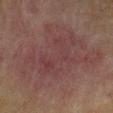Clinical impression:
This lesion was catalogued during total-body skin photography and was not selected for biopsy.
Acquisition and patient details:
The tile uses cross-polarized illumination. On the right upper arm. A roughly 15 mm field-of-view crop from a total-body skin photograph. The lesion's longest dimension is about 11.5 mm. The lesion-visualizer software estimated an area of roughly 60 mm², an outline eccentricity of about 0.75 (0 = round, 1 = elongated), and a shape-asymmetry score of about 0.35 (0 = symmetric). The software also gave an average lesion color of about L≈32 a*≈17 b*≈16 (CIELAB), about 5 CIELAB-L* units darker than the surrounding skin, and a normalized lesion–skin contrast near 5.5. The software also gave a color-variation rating of about 3.5/10 and peripheral color asymmetry of about 1.5. A male subject aged approximately 70.